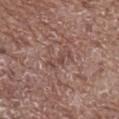Findings:
- workup · imaged on a skin check; not biopsied
- subject · male, aged around 70
- body site · the front of the torso
- automated metrics · an area of roughly 4 mm², an eccentricity of roughly 0.9, and a symmetry-axis asymmetry near 0.45; lesion-presence confidence of about 60/100
- acquisition · ~15 mm crop, total-body skin-cancer survey
- diameter · ~3.5 mm (longest diameter)
- illumination · white-light